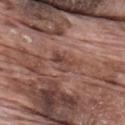follow-up: catalogued during a skin exam; not biopsied | subject: male, aged approximately 70 | imaging modality: ~15 mm crop, total-body skin-cancer survey | image-analysis metrics: an area of roughly 4 mm² and two-axis asymmetry of about 0.35; a normalized border contrast of about 6.5; border irregularity of about 4 on a 0–10 scale, a within-lesion color-variation index near 5/10, and radial color variation of about 2; a nevus-likeness score of about 0/100 and a lesion-detection confidence of about 95/100 | tile lighting: white-light illumination | lesion diameter: about 2.5 mm | site: the mid back.